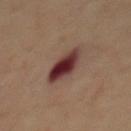Imaged during a routine full-body skin examination; the lesion was not biopsied and no histopathology is available. A region of skin cropped from a whole-body photographic capture, roughly 15 mm wide. The lesion is located on the front of the torso. A male subject, roughly 70 years of age. Approximately 4.5 mm at its widest. This is a cross-polarized tile.